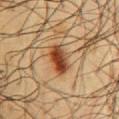Recorded during total-body skin imaging; not selected for excision or biopsy.
On the chest.
This image is a 15 mm lesion crop taken from a total-body photograph.
The lesion's longest dimension is about 4 mm.
An algorithmic analysis of the crop reported a footprint of about 7.5 mm², an eccentricity of roughly 0.85, and two-axis asymmetry of about 0.2. The software also gave a classifier nevus-likeness of about 100/100 and a detector confidence of about 100 out of 100 that the crop contains a lesion.
A male patient, approximately 60 years of age.